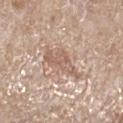Part of a total-body skin-imaging series; this lesion was reviewed on a skin check and was not flagged for biopsy.
The total-body-photography lesion software estimated a lesion area of about 9 mm², a shape eccentricity near 0.9, and a shape-asymmetry score of about 0.4 (0 = symmetric). The software also gave a border-irregularity rating of about 5/10, a color-variation rating of about 2.5/10, and radial color variation of about 1. And it measured lesion-presence confidence of about 80/100.
Captured under white-light illumination.
A female patient, approximately 70 years of age.
The lesion is on the right lower leg.
A roughly 15 mm field-of-view crop from a total-body skin photograph.